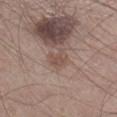This lesion was catalogued during total-body skin photography and was not selected for biopsy.
On the right lower leg.
A 15 mm close-up tile from a total-body photography series done for melanoma screening.
A male patient, aged 43 to 47.